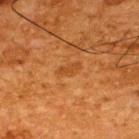Recorded during total-body skin imaging; not selected for excision or biopsy.
Automated tile analysis of the lesion measured an eccentricity of roughly 0.85 and a shape-asymmetry score of about 0.3 (0 = symmetric). It also reported an average lesion color of about L≈40 a*≈24 b*≈38 (CIELAB), a lesion–skin lightness drop of about 5, and a normalized border contrast of about 5. It also reported a border-irregularity index near 3/10 and a within-lesion color-variation index near 1/10.
The subject is a male aged around 65.
The lesion is on the upper back.
Imaged with cross-polarized lighting.
Longest diameter approximately 2.5 mm.
A 15 mm close-up extracted from a 3D total-body photography capture.